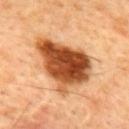Recorded during total-body skin imaging; not selected for excision or biopsy. A region of skin cropped from a whole-body photographic capture, roughly 15 mm wide. The lesion is located on the mid back. The tile uses cross-polarized illumination. A male subject aged 43 to 47. An algorithmic analysis of the crop reported a footprint of about 27 mm², an outline eccentricity of about 0.75 (0 = round, 1 = elongated), and two-axis asymmetry of about 0.35. And it measured about 22 CIELAB-L* units darker than the surrounding skin and a normalized border contrast of about 14.